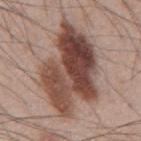{
  "biopsy_status": "not biopsied; imaged during a skin examination",
  "patient": {
    "sex": "male",
    "age_approx": 45
  },
  "site": "mid back",
  "image": {
    "source": "total-body photography crop",
    "field_of_view_mm": 15
  },
  "lesion_size": {
    "long_diameter_mm_approx": 10.5
  },
  "lighting": "white-light"
}